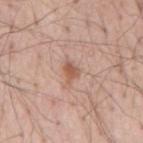| key | value |
|---|---|
| biopsy status | catalogued during a skin exam; not biopsied |
| patient | male, approximately 55 years of age |
| image source | 15 mm crop, total-body photography |
| site | the mid back |
| tile lighting | white-light |
| TBP lesion metrics | a lesion color around L≈57 a*≈21 b*≈30 in CIELAB, roughly 10 lightness units darker than nearby skin, and a normalized lesion–skin contrast near 7; a nevus-likeness score of about 55/100 and a lesion-detection confidence of about 100/100 |
| diameter | ≈2.5 mm |